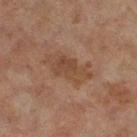{
  "biopsy_status": "not biopsied; imaged during a skin examination",
  "image": {
    "source": "total-body photography crop",
    "field_of_view_mm": 15
  },
  "lesion_size": {
    "long_diameter_mm_approx": 5.5
  },
  "patient": {
    "sex": "female",
    "age_approx": 60
  },
  "site": "right thigh"
}